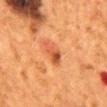Measured at roughly 3.5 mm in maximum diameter. From the back. A female subject, approximately 50 years of age. A 15 mm close-up extracted from a 3D total-body photography capture.